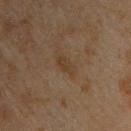This lesion was catalogued during total-body skin photography and was not selected for biopsy. A region of skin cropped from a whole-body photographic capture, roughly 15 mm wide. On the chest. A male subject about 45 years old.